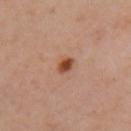  biopsy_status: not biopsied; imaged during a skin examination
  lesion_size:
    long_diameter_mm_approx: 2.0
  image:
    source: total-body photography crop
    field_of_view_mm: 15
  site: arm
  lighting: cross-polarized
  patient:
    sex: female
    age_approx: 40
  automated_metrics:
    eccentricity: 0.65
    shape_asymmetry: 0.2
    border_irregularity_0_10: 1.5
    peripheral_color_asymmetry: 2.0
    lesion_detection_confidence_0_100: 100